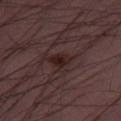{
  "biopsy_status": "not biopsied; imaged during a skin examination",
  "site": "right thigh",
  "lesion_size": {
    "long_diameter_mm_approx": 3.0
  },
  "automated_metrics": {
    "color_variation_0_10": 3.0,
    "peripheral_color_asymmetry": 1.0,
    "lesion_detection_confidence_0_100": 100
  },
  "lighting": "white-light",
  "patient": {
    "sex": "male",
    "age_approx": 50
  },
  "image": {
    "source": "total-body photography crop",
    "field_of_view_mm": 15
  }
}